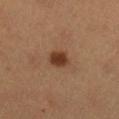Assessment: This lesion was catalogued during total-body skin photography and was not selected for biopsy. Clinical summary: The tile uses cross-polarized illumination. A female subject about 40 years old. A 15 mm close-up tile from a total-body photography series done for melanoma screening. The lesion is on the leg. The lesion's longest dimension is about 3 mm.